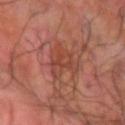Findings:
– workup: no biopsy performed (imaged during a skin exam)
– image source: ~15 mm crop, total-body skin-cancer survey
– lesion diameter: about 4.5 mm
– illumination: cross-polarized illumination
– subject: male, aged 58–62
– anatomic site: the left forearm